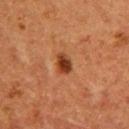Longest diameter approximately 3 mm.
The lesion is on the upper back.
The patient is a female aged around 40.
A lesion tile, about 15 mm wide, cut from a 3D total-body photograph.
This is a cross-polarized tile.